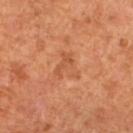{
  "biopsy_status": "not biopsied; imaged during a skin examination",
  "site": "left lower leg",
  "lighting": "cross-polarized",
  "image": {
    "source": "total-body photography crop",
    "field_of_view_mm": 15
  },
  "patient": {
    "sex": "female",
    "age_approx": 65
  },
  "lesion_size": {
    "long_diameter_mm_approx": 3.5
  }
}